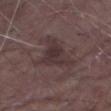The lesion was tiled from a total-body skin photograph and was not biopsied. The tile uses white-light illumination. A male subject, aged around 65. A region of skin cropped from a whole-body photographic capture, roughly 15 mm wide. From the arm. Approximately 5.5 mm at its widest.